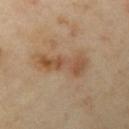workup — total-body-photography surveillance lesion; no biopsy
TBP lesion metrics — a lesion area of about 11 mm², an outline eccentricity of about 0.95 (0 = round, 1 = elongated), and two-axis asymmetry of about 0.35
imaging modality — total-body-photography crop, ~15 mm field of view
patient — female, approximately 40 years of age
lesion diameter — about 6 mm
tile lighting — cross-polarized
site — the left upper arm